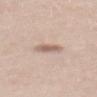site: back
image:
  source: total-body photography crop
  field_of_view_mm: 15
lighting: white-light
patient:
  sex: male
  age_approx: 40